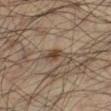Case summary:
• workup: imaged on a skin check; not biopsied
• site: the left lower leg
• diameter: about 3.5 mm
• subject: male, in their mid-30s
• image: total-body-photography crop, ~15 mm field of view
• tile lighting: cross-polarized